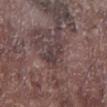Q: Was this lesion biopsied?
A: imaged on a skin check; not biopsied
Q: Illumination type?
A: white-light
Q: Where on the body is the lesion?
A: the left lower leg
Q: What is the lesion's diameter?
A: about 3.5 mm
Q: What did automated image analysis measure?
A: a shape-asymmetry score of about 0.35 (0 = symmetric); an automated nevus-likeness rating near 0 out of 100 and lesion-presence confidence of about 55/100
Q: What is the imaging modality?
A: 15 mm crop, total-body photography
Q: Patient demographics?
A: male, about 75 years old